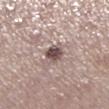The lesion was tiled from a total-body skin photograph and was not biopsied. A 15 mm close-up tile from a total-body photography series done for melanoma screening. A female subject aged approximately 65. About 3 mm across. From the right lower leg. The tile uses white-light illumination.